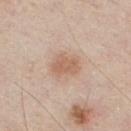<case>
<biopsy_status>not biopsied; imaged during a skin examination</biopsy_status>
</case>